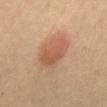Context: This image is a 15 mm lesion crop taken from a total-body photograph. A subject in their 60s. Located on the mid back.